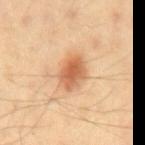Clinical summary: About 4 mm across. This is a cross-polarized tile. The lesion-visualizer software estimated a lesion area of about 9.5 mm², an outline eccentricity of about 0.65 (0 = round, 1 = elongated), and a symmetry-axis asymmetry near 0.15. And it measured a mean CIELAB color near L≈63 a*≈24 b*≈39 and roughly 13 lightness units darker than nearby skin. The software also gave border irregularity of about 1.5 on a 0–10 scale, a color-variation rating of about 5/10, and a peripheral color-asymmetry measure near 1.5. The software also gave a classifier nevus-likeness of about 95/100 and lesion-presence confidence of about 100/100. The subject is a male roughly 40 years of age. A 15 mm close-up tile from a total-body photography series done for melanoma screening. The lesion is located on the mid back.